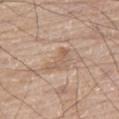Imaged during a routine full-body skin examination; the lesion was not biopsied and no histopathology is available.
About 3.5 mm across.
A male patient roughly 80 years of age.
An algorithmic analysis of the crop reported a mean CIELAB color near L≈58 a*≈17 b*≈30 and roughly 7 lightness units darker than nearby skin. It also reported a classifier nevus-likeness of about 0/100 and a lesion-detection confidence of about 95/100.
Cropped from a whole-body photographic skin survey; the tile spans about 15 mm.
Located on the leg.